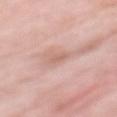subject: female, roughly 60 years of age | site: the mid back | tile lighting: white-light illumination | TBP lesion metrics: a footprint of about 2 mm², a shape eccentricity near 0.95, and a shape-asymmetry score of about 0.3 (0 = symmetric); a lesion color around L≈63 a*≈21 b*≈27 in CIELAB; a classifier nevus-likeness of about 0/100 | size: about 2.5 mm | acquisition: ~15 mm tile from a whole-body skin photo.